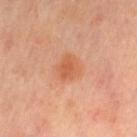| key | value |
|---|---|
| biopsy status | total-body-photography surveillance lesion; no biopsy |
| patient | female, aged 58 to 62 |
| image-analysis metrics | a classifier nevus-likeness of about 75/100 and lesion-presence confidence of about 100/100 |
| body site | the lower back |
| lesion diameter | ≈3 mm |
| image source | 15 mm crop, total-body photography |
| lighting | cross-polarized |